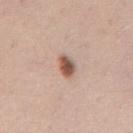  biopsy_status: not biopsied; imaged during a skin examination
  image:
    source: total-body photography crop
    field_of_view_mm: 15
  patient:
    sex: male
    age_approx: 35
  site: mid back